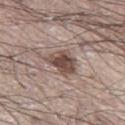Findings:
- workup · imaged on a skin check; not biopsied
- lesion size · ~4 mm (longest diameter)
- imaging modality · ~15 mm crop, total-body skin-cancer survey
- patient · male, in their 70s
- automated lesion analysis · a lesion area of about 9 mm², a shape eccentricity near 0.65, and two-axis asymmetry of about 0.3; an average lesion color of about L≈48 a*≈16 b*≈22 (CIELAB) and a normalized border contrast of about 9.5; a color-variation rating of about 5/10 and radial color variation of about 1.5; a nevus-likeness score of about 95/100 and a lesion-detection confidence of about 100/100
- location · the leg